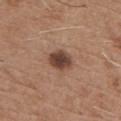Notes:
• biopsy status — no biopsy performed (imaged during a skin exam)
• body site — the chest
• imaging modality — total-body-photography crop, ~15 mm field of view
• patient — male, approximately 65 years of age